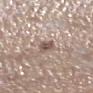Background: The lesion's longest dimension is about 2.5 mm. A 15 mm crop from a total-body photograph taken for skin-cancer surveillance. On the right lower leg. A female subject about 65 years old. Imaged with white-light lighting.